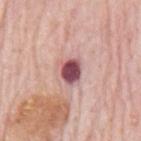No biopsy was performed on this lesion — it was imaged during a full skin examination and was not determined to be concerning. Located on the mid back. A male subject aged around 80. Cropped from a total-body skin-imaging series; the visible field is about 15 mm.